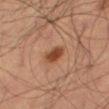No biopsy was performed on this lesion — it was imaged during a full skin examination and was not determined to be concerning. Imaged with cross-polarized lighting. A male subject, roughly 65 years of age. The lesion is located on the left thigh. This image is a 15 mm lesion crop taken from a total-body photograph.